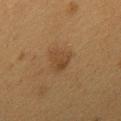biopsy_status: not biopsied; imaged during a skin examination
lighting: cross-polarized
patient:
  sex: female
  age_approx: 50
automated_metrics:
  cielab_L: 35
  cielab_a: 14
  cielab_b: 28
  vs_skin_contrast_norm: 5.5
image:
  source: total-body photography crop
  field_of_view_mm: 15
site: mid back